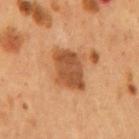Q: Is there a histopathology result?
A: no biopsy performed (imaged during a skin exam)
Q: Lesion location?
A: the back
Q: Illumination type?
A: cross-polarized
Q: Patient demographics?
A: male, aged approximately 55
Q: What kind of image is this?
A: ~15 mm crop, total-body skin-cancer survey